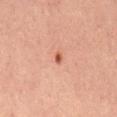Captured during whole-body skin photography for melanoma surveillance; the lesion was not biopsied. Longest diameter approximately 1.5 mm. This is a cross-polarized tile. A lesion tile, about 15 mm wide, cut from a 3D total-body photograph. The lesion is located on the mid back. The subject is a male roughly 30 years of age. Automated image analysis of the tile measured border irregularity of about 3 on a 0–10 scale, a within-lesion color-variation index near 0/10, and radial color variation of about 0. It also reported a classifier nevus-likeness of about 60/100 and a lesion-detection confidence of about 100/100.